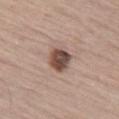| field | value |
|---|---|
| notes | total-body-photography surveillance lesion; no biopsy |
| patient | male, in their mid- to late 60s |
| tile lighting | white-light illumination |
| site | the left thigh |
| diameter | ~3 mm (longest diameter) |
| image-analysis metrics | an average lesion color of about L≈46 a*≈19 b*≈24 (CIELAB) and a lesion-to-skin contrast of about 12 (normalized; higher = more distinct); an automated nevus-likeness rating near 75 out of 100 and a lesion-detection confidence of about 100/100 |
| image | 15 mm crop, total-body photography |